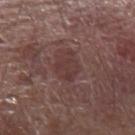Impression: This lesion was catalogued during total-body skin photography and was not selected for biopsy. Image and clinical context: From the left forearm. A male patient, aged 73–77. Captured under white-light illumination. The recorded lesion diameter is about 4 mm. A close-up tile cropped from a whole-body skin photograph, about 15 mm across.